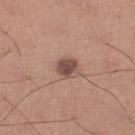Impression:
This lesion was catalogued during total-body skin photography and was not selected for biopsy.
Context:
The lesion is on the right lower leg. A male subject, aged approximately 60. A close-up tile cropped from a whole-body skin photograph, about 15 mm across.